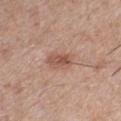Captured during whole-body skin photography for melanoma surveillance; the lesion was not biopsied.
A male patient roughly 30 years of age.
The lesion is located on the chest.
The lesion's longest dimension is about 3.5 mm.
A 15 mm crop from a total-body photograph taken for skin-cancer surveillance.
Imaged with white-light lighting.